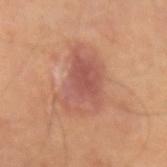– biopsy status · no biopsy performed (imaged during a skin exam)
– lesion diameter · ≈6.5 mm
– automated metrics · a footprint of about 23 mm² and an eccentricity of roughly 0.8; an average lesion color of about L≈53 a*≈24 b*≈28 (CIELAB), a lesion–skin lightness drop of about 9, and a lesion-to-skin contrast of about 6.5 (normalized; higher = more distinct); border irregularity of about 3.5 on a 0–10 scale, internal color variation of about 5 on a 0–10 scale, and radial color variation of about 1.5
– tile lighting · cross-polarized
– image · ~15 mm tile from a whole-body skin photo
– subject · male, approximately 85 years of age or older
– body site · the left forearm